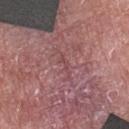A female subject about 60 years old.
A roughly 15 mm field-of-view crop from a total-body skin photograph.
On the left forearm.
Captured under white-light illumination.
Approximately 2.5 mm at its widest.